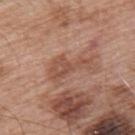| feature | finding |
|---|---|
| body site | the upper back |
| acquisition | total-body-photography crop, ~15 mm field of view |
| patient | male, aged around 55 |